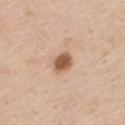Q: Is there a histopathology result?
A: imaged on a skin check; not biopsied
Q: Lesion location?
A: the chest
Q: What is the lesion's diameter?
A: ≈2.5 mm
Q: Automated lesion metrics?
A: a footprint of about 4.5 mm² and an outline eccentricity of about 0.65 (0 = round, 1 = elongated); an average lesion color of about L≈56 a*≈21 b*≈33 (CIELAB) and a lesion-to-skin contrast of about 10 (normalized; higher = more distinct); a nevus-likeness score of about 95/100 and lesion-presence confidence of about 100/100
Q: What kind of image is this?
A: ~15 mm tile from a whole-body skin photo
Q: What lighting was used for the tile?
A: white-light
Q: Patient demographics?
A: female, about 55 years old